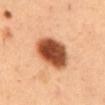Captured during whole-body skin photography for melanoma surveillance; the lesion was not biopsied. The tile uses cross-polarized illumination. A male subject, aged approximately 50. Automated tile analysis of the lesion measured a mean CIELAB color near L≈51 a*≈28 b*≈37, a lesion–skin lightness drop of about 23, and a normalized border contrast of about 14. And it measured border irregularity of about 1 on a 0–10 scale and a within-lesion color-variation index near 6.5/10. And it measured a classifier nevus-likeness of about 100/100 and lesion-presence confidence of about 100/100. On the abdomen. Cropped from a total-body skin-imaging series; the visible field is about 15 mm. About 5 mm across.